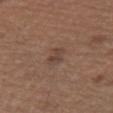{
  "biopsy_status": "not biopsied; imaged during a skin examination",
  "image": {
    "source": "total-body photography crop",
    "field_of_view_mm": 15
  },
  "lesion_size": {
    "long_diameter_mm_approx": 2.5
  },
  "patient": {
    "sex": "female",
    "age_approx": 65
  },
  "automated_metrics": {
    "area_mm2_approx": 4.0,
    "eccentricity": 0.75,
    "cielab_L": 42,
    "cielab_a": 17,
    "cielab_b": 24,
    "vs_skin_darker_L": 7.0,
    "vs_skin_contrast_norm": 6.0,
    "nevus_likeness_0_100": 0
  },
  "site": "right upper arm"
}